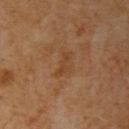Impression:
No biopsy was performed on this lesion — it was imaged during a full skin examination and was not determined to be concerning.
Background:
A lesion tile, about 15 mm wide, cut from a 3D total-body photograph. The tile uses cross-polarized illumination. From the arm. A male patient, about 60 years old. Longest diameter approximately 3 mm.